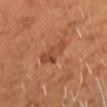The lesion was photographed on a routine skin check and not biopsied; there is no pathology result. Located on the head or neck. A male patient roughly 60 years of age. Cropped from a total-body skin-imaging series; the visible field is about 15 mm.